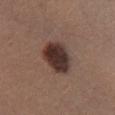follow-up — no biopsy performed (imaged during a skin exam); subject — male, aged 33 to 37; location — the left lower leg; acquisition — total-body-photography crop, ~15 mm field of view.